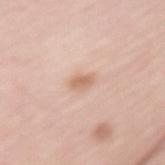Findings:
• workup: catalogued during a skin exam; not biopsied
• patient: female, roughly 65 years of age
• automated lesion analysis: a lesion area of about 3 mm², an eccentricity of roughly 0.85, and a symmetry-axis asymmetry near 0.3; a lesion color around L≈66 a*≈20 b*≈31 in CIELAB, roughly 10 lightness units darker than nearby skin, and a normalized lesion–skin contrast near 6.5; a border-irregularity index near 2.5/10, internal color variation of about 2 on a 0–10 scale, and a peripheral color-asymmetry measure near 0.5; an automated nevus-likeness rating near 65 out of 100 and a detector confidence of about 100 out of 100 that the crop contains a lesion
• lesion diameter: ≈2.5 mm
• acquisition: ~15 mm crop, total-body skin-cancer survey
• site: the left forearm
• tile lighting: white-light